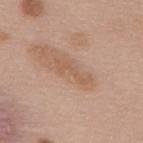No biopsy was performed on this lesion — it was imaged during a full skin examination and was not determined to be concerning. A female patient, approximately 60 years of age. Automated image analysis of the tile measured an area of roughly 3.5 mm² and an outline eccentricity of about 0.95 (0 = round, 1 = elongated). The analysis additionally found border irregularity of about 8.5 on a 0–10 scale and a color-variation rating of about 0.5/10. The lesion's longest dimension is about 4.5 mm. Captured under white-light illumination. From the back. A 15 mm close-up tile from a total-body photography series done for melanoma screening.